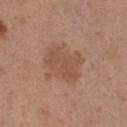biopsy_status: not biopsied; imaged during a skin examination
lighting: white-light
patient:
  sex: female
  age_approx: 30
site: right thigh
image:
  source: total-body photography crop
  field_of_view_mm: 15
lesion_size:
  long_diameter_mm_approx: 4.5